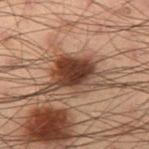{
  "image": {
    "source": "total-body photography crop",
    "field_of_view_mm": 15
  },
  "site": "left thigh",
  "patient": {
    "sex": "male",
    "age_approx": 55
  }
}